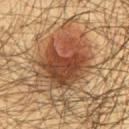Assessment:
The lesion was tiled from a total-body skin photograph and was not biopsied.
Acquisition and patient details:
Cropped from a whole-body photographic skin survey; the tile spans about 15 mm. From the lower back. A male subject, in their mid- to late 40s. Measured at roughly 6 mm in maximum diameter. Captured under cross-polarized illumination.